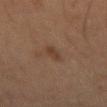Captured during whole-body skin photography for melanoma surveillance; the lesion was not biopsied.
A male subject, in their mid- to late 60s.
A 15 mm crop from a total-body photograph taken for skin-cancer surveillance.
On the back.